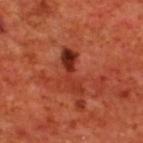Captured during whole-body skin photography for melanoma surveillance; the lesion was not biopsied.
Measured at roughly 5.5 mm in maximum diameter.
The total-body-photography lesion software estimated a lesion area of about 8 mm², a shape eccentricity near 0.95, and a shape-asymmetry score of about 0.35 (0 = symmetric). It also reported border irregularity of about 5.5 on a 0–10 scale, a color-variation rating of about 9/10, and peripheral color asymmetry of about 3. The analysis additionally found a classifier nevus-likeness of about 0/100.
From the upper back.
The patient is a male aged around 70.
A region of skin cropped from a whole-body photographic capture, roughly 15 mm wide.